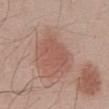Imaged during a routine full-body skin examination; the lesion was not biopsied and no histopathology is available.
A male subject, in their 50s.
The lesion is located on the abdomen.
A 15 mm close-up tile from a total-body photography series done for melanoma screening.
This is a white-light tile.
About 4.5 mm across.